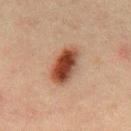{"biopsy_status": "not biopsied; imaged during a skin examination", "image": {"source": "total-body photography crop", "field_of_view_mm": 15}, "site": "mid back", "patient": {"sex": "male", "age_approx": 50}}